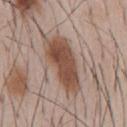* workup — catalogued during a skin exam; not biopsied
* imaging modality — total-body-photography crop, ~15 mm field of view
* image-analysis metrics — an area of roughly 19 mm², a shape eccentricity near 0.9, and a shape-asymmetry score of about 0.25 (0 = symmetric)
* subject — male, in their mid-50s